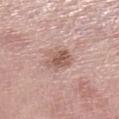Notes:
* workup — no biopsy performed (imaged during a skin exam)
* lighting — white-light illumination
* site — the left lower leg
* imaging modality — 15 mm crop, total-body photography
* size — about 3.5 mm
* subject — female, aged approximately 70
* automated lesion analysis — a border-irregularity rating of about 2.5/10 and internal color variation of about 3 on a 0–10 scale